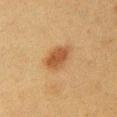Q: Was a biopsy performed?
A: imaged on a skin check; not biopsied
Q: Automated lesion metrics?
A: a footprint of about 9 mm², an eccentricity of roughly 0.75, and a symmetry-axis asymmetry near 0.2; a lesion color around L≈44 a*≈18 b*≈33 in CIELAB, a lesion–skin lightness drop of about 9, and a normalized border contrast of about 7.5; a border-irregularity index near 2/10 and a color-variation rating of about 3/10; an automated nevus-likeness rating near 100 out of 100 and a lesion-detection confidence of about 100/100
Q: How large is the lesion?
A: about 4 mm
Q: What are the patient's age and sex?
A: female, about 40 years old
Q: What kind of image is this?
A: ~15 mm crop, total-body skin-cancer survey
Q: How was the tile lit?
A: cross-polarized illumination
Q: Lesion location?
A: the chest